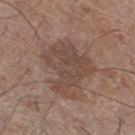workup — total-body-photography surveillance lesion; no biopsy
body site — the leg
diameter — about 6 mm
patient — male, aged 58–62
lighting — white-light
acquisition — ~15 mm crop, total-body skin-cancer survey